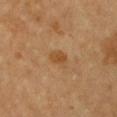Recorded during total-body skin imaging; not selected for excision or biopsy. A 15 mm crop from a total-body photograph taken for skin-cancer surveillance. The tile uses cross-polarized illumination. The subject is a female aged around 65. The lesion is located on the chest.